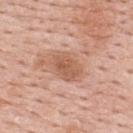biopsy_status: not biopsied; imaged during a skin examination
patient:
  sex: male
  age_approx: 55
automated_metrics:
  shape_asymmetry: 0.2
  vs_skin_darker_L: 9.0
  vs_skin_contrast_norm: 6.5
lesion_size:
  long_diameter_mm_approx: 4.0
image:
  source: total-body photography crop
  field_of_view_mm: 15
site: upper back
lighting: white-light